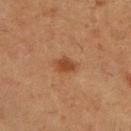<case>
<biopsy_status>not biopsied; imaged during a skin examination</biopsy_status>
<lesion_size>
  <long_diameter_mm_approx>2.5</long_diameter_mm_approx>
</lesion_size>
<image>
  <source>total-body photography crop</source>
  <field_of_view_mm>15</field_of_view_mm>
</image>
<lighting>cross-polarized</lighting>
<site>right lower leg</site>
<patient>
  <sex>female</sex>
  <age_approx>50</age_approx>
</patient>
</case>